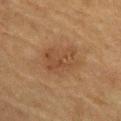Imaged during a routine full-body skin examination; the lesion was not biopsied and no histopathology is available. From the abdomen. A roughly 15 mm field-of-view crop from a total-body skin photograph. A male patient, aged around 85 or older. An algorithmic analysis of the crop reported an automated nevus-likeness rating near 20 out of 100 and a detector confidence of about 100 out of 100 that the crop contains a lesion.